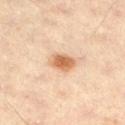Imaged during a routine full-body skin examination; the lesion was not biopsied and no histopathology is available.
Approximately 3 mm at its widest.
A male patient, in their 60s.
Cropped from a total-body skin-imaging series; the visible field is about 15 mm.
This is a cross-polarized tile.
The lesion is on the right thigh.
Automated tile analysis of the lesion measured an average lesion color of about L≈62 a*≈20 b*≈36 (CIELAB), a lesion–skin lightness drop of about 12, and a normalized border contrast of about 8.5. The analysis additionally found a border-irregularity rating of about 1.5/10, a within-lesion color-variation index near 3.5/10, and a peripheral color-asymmetry measure near 1. It also reported an automated nevus-likeness rating near 100 out of 100.